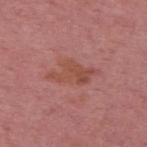No biopsy was performed on this lesion — it was imaged during a full skin examination and was not determined to be concerning.
A male patient in their mid-50s.
Cropped from a whole-body photographic skin survey; the tile spans about 15 mm.
The lesion is on the upper back.
The lesion's longest dimension is about 5.5 mm.
Imaged with white-light lighting.
An algorithmic analysis of the crop reported a mean CIELAB color near L≈49 a*≈26 b*≈27 and a lesion-to-skin contrast of about 6.5 (normalized; higher = more distinct).